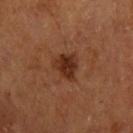notes: imaged on a skin check; not biopsied
anatomic site: the left upper arm
subject: male, roughly 65 years of age
illumination: cross-polarized
size: about 3.5 mm
automated metrics: a lesion area of about 7 mm² and a symmetry-axis asymmetry near 0.35; a mean CIELAB color near L≈29 a*≈22 b*≈29, a lesion–skin lightness drop of about 10, and a normalized lesion–skin contrast near 9.5; an automated nevus-likeness rating near 85 out of 100 and a detector confidence of about 100 out of 100 that the crop contains a lesion
image source: 15 mm crop, total-body photography